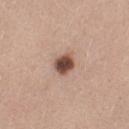Impression:
No biopsy was performed on this lesion — it was imaged during a full skin examination and was not determined to be concerning.
Context:
From the leg. Cropped from a whole-body photographic skin survey; the tile spans about 15 mm. The patient is a female roughly 45 years of age.